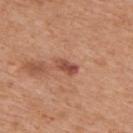A male patient, aged 68–72. The lesion is located on the upper back. A 15 mm close-up tile from a total-body photography series done for melanoma screening.